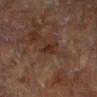Q: Is there a histopathology result?
A: catalogued during a skin exam; not biopsied
Q: Automated lesion metrics?
A: a nevus-likeness score of about 0/100 and a lesion-detection confidence of about 100/100
Q: What are the patient's age and sex?
A: male, roughly 65 years of age
Q: What is the lesion's diameter?
A: ≈2.5 mm
Q: Illumination type?
A: cross-polarized
Q: What kind of image is this?
A: ~15 mm tile from a whole-body skin photo
Q: Where on the body is the lesion?
A: the left forearm